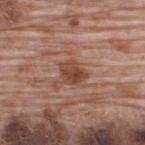This lesion was catalogued during total-body skin photography and was not selected for biopsy. The recorded lesion diameter is about 3 mm. The lesion is on the upper back. A lesion tile, about 15 mm wide, cut from a 3D total-body photograph. The patient is a male about 70 years old.